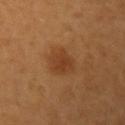Impression: The lesion was tiled from a total-body skin photograph and was not biopsied. Acquisition and patient details: A 15 mm close-up tile from a total-body photography series done for melanoma screening. From the left upper arm. A female subject aged 43 to 47.